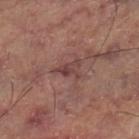• biopsy status: catalogued during a skin exam; not biopsied
• location: the left thigh
• tile lighting: cross-polarized
• imaging modality: 15 mm crop, total-body photography
• lesion size: ≈3.5 mm